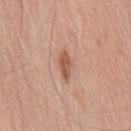biopsy status — total-body-photography surveillance lesion; no biopsy | patient — male, approximately 75 years of age | body site — the leg | image — total-body-photography crop, ~15 mm field of view | image-analysis metrics — a lesion color around L≈55 a*≈24 b*≈30 in CIELAB, about 12 CIELAB-L* units darker than the surrounding skin, and a normalized border contrast of about 8; border irregularity of about 3 on a 0–10 scale, internal color variation of about 1.5 on a 0–10 scale, and a peripheral color-asymmetry measure near 0.5; a nevus-likeness score of about 70/100 and a lesion-detection confidence of about 100/100 | lesion size — ≈3.5 mm.